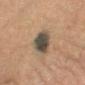Case summary:
- workup: no biopsy performed (imaged during a skin exam)
- size: about 4.5 mm
- lighting: cross-polarized
- automated lesion analysis: a mean CIELAB color near L≈40 a*≈7 b*≈20, roughly 15 lightness units darker than nearby skin, and a normalized border contrast of about 13; a border-irregularity rating of about 2/10, internal color variation of about 5 on a 0–10 scale, and peripheral color asymmetry of about 1.5
- subject: male, in their mid-50s
- acquisition: 15 mm crop, total-body photography
- site: the arm